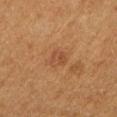workup=total-body-photography surveillance lesion; no biopsy | patient=female, aged 38 to 42 | acquisition=total-body-photography crop, ~15 mm field of view | anatomic site=the arm.